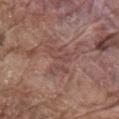Notes:
– biopsy status: imaged on a skin check; not biopsied
– acquisition: ~15 mm tile from a whole-body skin photo
– lesion size: ~3.5 mm (longest diameter)
– anatomic site: the mid back
– subject: male, approximately 80 years of age
– tile lighting: white-light